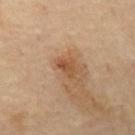Findings:
* notes — no biopsy performed (imaged during a skin exam)
* location — the mid back
* lighting — cross-polarized illumination
* image source — total-body-photography crop, ~15 mm field of view
* lesion diameter — ~2.5 mm (longest diameter)
* automated metrics — about 9 CIELAB-L* units darker than the surrounding skin and a normalized border contrast of about 7.5; border irregularity of about 3 on a 0–10 scale, internal color variation of about 1.5 on a 0–10 scale, and radial color variation of about 0.5
* subject — male, in their 70s